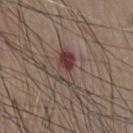Part of a total-body skin-imaging series; this lesion was reviewed on a skin check and was not flagged for biopsy. The total-body-photography lesion software estimated a mean CIELAB color near L≈39 a*≈19 b*≈18 and a normalized border contrast of about 9. The analysis additionally found internal color variation of about 4.5 on a 0–10 scale and radial color variation of about 2. The tile uses white-light illumination. Cropped from a total-body skin-imaging series; the visible field is about 15 mm. The subject is a male aged around 80. Located on the chest. Measured at roughly 4 mm in maximum diameter.